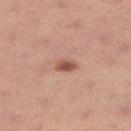Q: Was this lesion biopsied?
A: imaged on a skin check; not biopsied
Q: Who is the patient?
A: female, in their mid-20s
Q: What is the anatomic site?
A: the leg
Q: What is the imaging modality?
A: ~15 mm crop, total-body skin-cancer survey